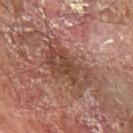Findings:
• workup · imaged on a skin check; not biopsied
• lesion diameter · about 6.5 mm
• automated metrics · a lesion area of about 17 mm² and a shape-asymmetry score of about 0.35 (0 = symmetric); a nevus-likeness score of about 0/100 and lesion-presence confidence of about 90/100
• imaging modality · ~15 mm tile from a whole-body skin photo
• site · the left forearm
• lighting · cross-polarized
• subject · male, aged 68–72